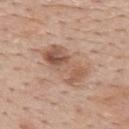Q: What are the patient's age and sex?
A: male, aged around 55
Q: What lighting was used for the tile?
A: white-light
Q: Lesion location?
A: the mid back
Q: What is the imaging modality?
A: 15 mm crop, total-body photography
Q: What is the lesion's diameter?
A: about 6 mm
Q: Automated lesion metrics?
A: a classifier nevus-likeness of about 30/100 and a detector confidence of about 100 out of 100 that the crop contains a lesion